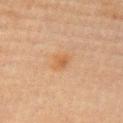Q: Was a biopsy performed?
A: total-body-photography surveillance lesion; no biopsy
Q: What is the anatomic site?
A: the left upper arm
Q: Who is the patient?
A: male, approximately 70 years of age
Q: What kind of image is this?
A: ~15 mm crop, total-body skin-cancer survey